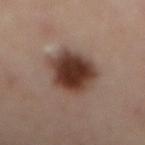Clinical summary:
A female patient, aged 58–62. A lesion tile, about 15 mm wide, cut from a 3D total-body photograph. From the mid back.